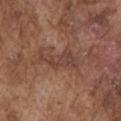diameter = ~5 mm (longest diameter); body site = the left upper arm; acquisition = ~15 mm tile from a whole-body skin photo; illumination = white-light illumination; patient = male, roughly 75 years of age.